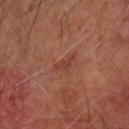Assessment: Captured during whole-body skin photography for melanoma surveillance; the lesion was not biopsied. Context: Longest diameter approximately 3 mm. The tile uses cross-polarized illumination. A 15 mm close-up tile from a total-body photography series done for melanoma screening. From the right forearm. A male patient aged 68 to 72.